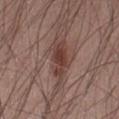Assessment: Part of a total-body skin-imaging series; this lesion was reviewed on a skin check and was not flagged for biopsy. Background: The lesion is on the right thigh. This is a white-light tile. A 15 mm crop from a total-body photograph taken for skin-cancer surveillance. A male patient aged around 55. An algorithmic analysis of the crop reported an area of roughly 8.5 mm², an eccentricity of roughly 0.9, and a symmetry-axis asymmetry near 0.35. It also reported an average lesion color of about L≈40 a*≈19 b*≈23 (CIELAB), a lesion–skin lightness drop of about 10, and a normalized border contrast of about 8.5. The software also gave a border-irregularity index near 5.5/10 and a peripheral color-asymmetry measure near 1. And it measured an automated nevus-likeness rating near 95 out of 100 and a lesion-detection confidence of about 100/100.